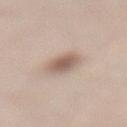Clinical impression:
This lesion was catalogued during total-body skin photography and was not selected for biopsy.
Context:
Automated image analysis of the tile measured an area of roughly 8 mm², an outline eccentricity of about 0.7 (0 = round, 1 = elongated), and a shape-asymmetry score of about 0.2 (0 = symmetric). And it measured a border-irregularity index near 2/10, internal color variation of about 4 on a 0–10 scale, and a peripheral color-asymmetry measure near 1. The analysis additionally found a nevus-likeness score of about 90/100 and a detector confidence of about 100 out of 100 that the crop contains a lesion. A female patient, aged 63–67. Cropped from a total-body skin-imaging series; the visible field is about 15 mm. On the lower back. Approximately 4 mm at its widest. Captured under white-light illumination.